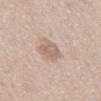Notes:
* biopsy status — no biopsy performed (imaged during a skin exam)
* acquisition — ~15 mm crop, total-body skin-cancer survey
* location — the abdomen
* diameter — ~3 mm (longest diameter)
* patient — male, roughly 60 years of age
* image-analysis metrics — an eccentricity of roughly 0.25 and a symmetry-axis asymmetry near 0.1; a border-irregularity index near 1.5/10, internal color variation of about 2.5 on a 0–10 scale, and radial color variation of about 1